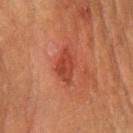Imaged during a routine full-body skin examination; the lesion was not biopsied and no histopathology is available.
Cropped from a total-body skin-imaging series; the visible field is about 15 mm.
The lesion is on the right forearm.
The lesion-visualizer software estimated an outline eccentricity of about 0.85 (0 = round, 1 = elongated) and a shape-asymmetry score of about 0.25 (0 = symmetric). The software also gave a border-irregularity rating of about 2.5/10 and a within-lesion color-variation index near 3/10. It also reported a classifier nevus-likeness of about 35/100 and a lesion-detection confidence of about 100/100.
A female patient aged around 80.
Longest diameter approximately 4 mm.